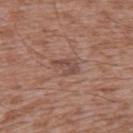{
  "lighting": "white-light",
  "automated_metrics": {
    "area_mm2_approx": 3.5,
    "cielab_L": 47,
    "cielab_a": 20,
    "cielab_b": 25,
    "vs_skin_darker_L": 8.0,
    "vs_skin_contrast_norm": 6.5,
    "border_irregularity_0_10": 6.5,
    "color_variation_0_10": 0.0,
    "peripheral_color_asymmetry": 0.0,
    "nevus_likeness_0_100": 0,
    "lesion_detection_confidence_0_100": 100
  },
  "patient": {
    "sex": "male",
    "age_approx": 45
  },
  "image": {
    "source": "total-body photography crop",
    "field_of_view_mm": 15
  },
  "lesion_size": {
    "long_diameter_mm_approx": 3.0
  },
  "site": "left upper arm"
}